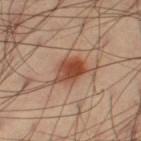The lesion was tiled from a total-body skin photograph and was not biopsied. A roughly 15 mm field-of-view crop from a total-body skin photograph. The lesion is located on the left thigh. The tile uses cross-polarized illumination. A male subject, aged around 40. Longest diameter approximately 4 mm.